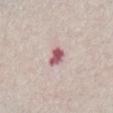<record>
  <biopsy_status>not biopsied; imaged during a skin examination</biopsy_status>
  <lighting>white-light</lighting>
  <lesion_size>
    <long_diameter_mm_approx>2.5</long_diameter_mm_approx>
  </lesion_size>
  <image>
    <source>total-body photography crop</source>
    <field_of_view_mm>15</field_of_view_mm>
  </image>
  <patient>
    <sex>male</sex>
    <age_approx>65</age_approx>
  </patient>
  <site>front of the torso</site>
</record>